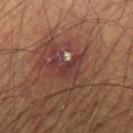follow-up — imaged on a skin check; not biopsied | lesion size — ~4 mm (longest diameter) | image-analysis metrics — a border-irregularity rating of about 5.5/10, a within-lesion color-variation index near 2.5/10, and a peripheral color-asymmetry measure near 0.5; an automated nevus-likeness rating near 0 out of 100 and lesion-presence confidence of about 70/100 | illumination — cross-polarized illumination | acquisition — ~15 mm tile from a whole-body skin photo | subject — male, aged around 60.